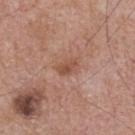Part of a total-body skin-imaging series; this lesion was reviewed on a skin check and was not flagged for biopsy. The recorded lesion diameter is about 2.5 mm. A male subject, in their mid-60s. The lesion-visualizer software estimated an average lesion color of about L≈51 a*≈22 b*≈29 (CIELAB), a lesion–skin lightness drop of about 9, and a normalized lesion–skin contrast near 6.5. And it measured a border-irregularity rating of about 2/10 and radial color variation of about 1. A roughly 15 mm field-of-view crop from a total-body skin photograph. Captured under white-light illumination. The lesion is located on the upper back.